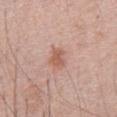workup: imaged on a skin check; not biopsied
illumination: white-light
location: the chest
image-analysis metrics: an average lesion color of about L≈58 a*≈22 b*≈29 (CIELAB), about 10 CIELAB-L* units darker than the surrounding skin, and a lesion-to-skin contrast of about 7 (normalized; higher = more distinct); a border-irregularity index near 3.5/10, internal color variation of about 2.5 on a 0–10 scale, and radial color variation of about 1; an automated nevus-likeness rating near 60 out of 100 and a lesion-detection confidence of about 100/100
patient: male, approximately 70 years of age
acquisition: total-body-photography crop, ~15 mm field of view
lesion diameter: ≈2.5 mm